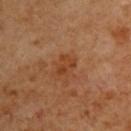No biopsy was performed on this lesion — it was imaged during a full skin examination and was not determined to be concerning. On the back. The subject is a male aged approximately 60. This is a cross-polarized tile. A 15 mm close-up extracted from a 3D total-body photography capture.